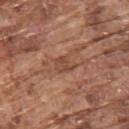{
  "biopsy_status": "not biopsied; imaged during a skin examination",
  "image": {
    "source": "total-body photography crop",
    "field_of_view_mm": 15
  },
  "lighting": "white-light",
  "patient": {
    "sex": "male",
    "age_approx": 75
  },
  "site": "upper back",
  "lesion_size": {
    "long_diameter_mm_approx": 3.0
  },
  "automated_metrics": {
    "area_mm2_approx": 4.0,
    "eccentricity": 0.7,
    "shape_asymmetry": 0.3,
    "cielab_L": 47,
    "cielab_a": 22,
    "cielab_b": 30,
    "vs_skin_contrast_norm": 6.0,
    "border_irregularity_0_10": 3.0,
    "color_variation_0_10": 2.0,
    "peripheral_color_asymmetry": 1.0,
    "nevus_likeness_0_100": 0,
    "lesion_detection_confidence_0_100": 95
  }
}